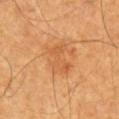  biopsy_status: not biopsied; imaged during a skin examination
  patient:
    sex: male
    age_approx: 60
  site: chest
  lighting: cross-polarized
  automated_metrics:
    area_mm2_approx: 7.0
    eccentricity: 0.75
    shape_asymmetry: 0.35
    cielab_L: 53
    cielab_a: 24
    cielab_b: 39
    vs_skin_darker_L: 6.0
    vs_skin_contrast_norm: 4.5
    nevus_likeness_0_100: 15
  lesion_size:
    long_diameter_mm_approx: 3.5
  image:
    source: total-body photography crop
    field_of_view_mm: 15